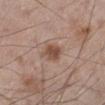<record>
  <biopsy_status>not biopsied; imaged during a skin examination</biopsy_status>
  <lesion_size>
    <long_diameter_mm_approx>2.5</long_diameter_mm_approx>
  </lesion_size>
  <lighting>white-light</lighting>
  <automated_metrics>
    <area_mm2_approx>4.5</area_mm2_approx>
    <eccentricity>0.65</eccentricity>
    <shape_asymmetry>0.25</shape_asymmetry>
  </automated_metrics>
  <patient>
    <sex>male</sex>
    <age_approx>60</age_approx>
  </patient>
  <image>
    <source>total-body photography crop</source>
    <field_of_view_mm>15</field_of_view_mm>
  </image>
  <site>left lower leg</site>
</record>